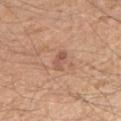This lesion was catalogued during total-body skin photography and was not selected for biopsy. Cropped from a total-body skin-imaging series; the visible field is about 15 mm. Approximately 3 mm at its widest. Located on the right upper arm. The subject is a male about 35 years old. Automated image analysis of the tile measured an average lesion color of about L≈55 a*≈24 b*≈29 (CIELAB), a lesion–skin lightness drop of about 9, and a lesion-to-skin contrast of about 6 (normalized; higher = more distinct). The tile uses white-light illumination.